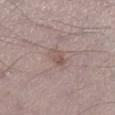{
  "patient": {
    "sex": "male",
    "age_approx": 70
  },
  "site": "leg",
  "automated_metrics": {
    "eccentricity": 0.55,
    "nevus_likeness_0_100": 20
  },
  "lesion_size": {
    "long_diameter_mm_approx": 2.5
  },
  "image": {
    "source": "total-body photography crop",
    "field_of_view_mm": 15
  },
  "lighting": "white-light"
}